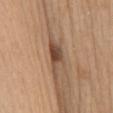Automated image analysis of the tile measured an average lesion color of about L≈48 a*≈18 b*≈29 (CIELAB) and about 13 CIELAB-L* units darker than the surrounding skin. The analysis additionally found border irregularity of about 5.5 on a 0–10 scale and a within-lesion color-variation index near 4.5/10. A 15 mm close-up tile from a total-body photography series done for melanoma screening. Imaged with white-light lighting. The lesion is located on the mid back. The patient is a female aged approximately 70.